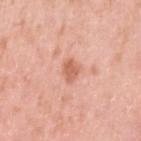Recorded during total-body skin imaging; not selected for excision or biopsy. The total-body-photography lesion software estimated an average lesion color of about L≈63 a*≈27 b*≈33 (CIELAB) and about 11 CIELAB-L* units darker than the surrounding skin. The analysis additionally found border irregularity of about 2.5 on a 0–10 scale, a within-lesion color-variation index near 1.5/10, and a peripheral color-asymmetry measure near 0.5. From the left upper arm. The lesion's longest dimension is about 3 mm. This is a white-light tile. A female patient, about 40 years old. This image is a 15 mm lesion crop taken from a total-body photograph.